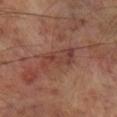follow-up = imaged on a skin check; not biopsied | diameter = ~4.5 mm (longest diameter) | body site = the leg | patient = male, aged 68 to 72 | illumination = cross-polarized | imaging modality = total-body-photography crop, ~15 mm field of view | automated metrics = a lesion area of about 8.5 mm², an eccentricity of roughly 0.85, and two-axis asymmetry of about 0.4; lesion-presence confidence of about 100/100.